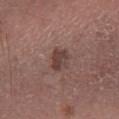Notes:
* imaging modality: ~15 mm tile from a whole-body skin photo
* diameter: ~3 mm (longest diameter)
* illumination: white-light
* site: the right lower leg
* patient: male, in their mid- to late 60s